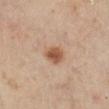<case>
  <biopsy_status>not biopsied; imaged during a skin examination</biopsy_status>
  <patient>
    <sex>female</sex>
    <age_approx>60</age_approx>
  </patient>
  <site>left lower leg</site>
  <lighting>cross-polarized</lighting>
  <image>
    <source>total-body photography crop</source>
    <field_of_view_mm>15</field_of_view_mm>
  </image>
</case>